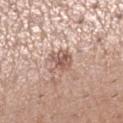biopsy_status: not biopsied; imaged during a skin examination
image:
  source: total-body photography crop
  field_of_view_mm: 15
lighting: white-light
site: right lower leg
lesion_size:
  long_diameter_mm_approx: 3.5
patient:
  sex: female
  age_approx: 30